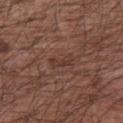Assessment: This lesion was catalogued during total-body skin photography and was not selected for biopsy. Background: Imaged with white-light lighting. A 15 mm close-up extracted from a 3D total-body photography capture. A male patient, aged 63 to 67. Located on the left upper arm. Automated image analysis of the tile measured a footprint of about 3 mm², an eccentricity of roughly 0.9, and a symmetry-axis asymmetry near 0.4.